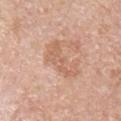Imaged during a routine full-body skin examination; the lesion was not biopsied and no histopathology is available.
The lesion is located on the arm.
Automated image analysis of the tile measured a border-irregularity index near 7/10 and peripheral color asymmetry of about 0.5. The software also gave a lesion-detection confidence of about 100/100.
Imaged with white-light lighting.
A lesion tile, about 15 mm wide, cut from a 3D total-body photograph.
Longest diameter approximately 5 mm.
A male patient, aged 73 to 77.